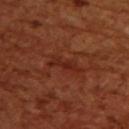Q: Where on the body is the lesion?
A: the upper back
Q: Automated lesion metrics?
A: a footprint of about 4.5 mm² and an eccentricity of roughly 0.95; border irregularity of about 6 on a 0–10 scale, a within-lesion color-variation index near 1/10, and peripheral color asymmetry of about 0; a nevus-likeness score of about 0/100 and lesion-presence confidence of about 90/100
Q: What kind of image is this?
A: total-body-photography crop, ~15 mm field of view
Q: Patient demographics?
A: female, aged 53–57
Q: How large is the lesion?
A: about 4 mm